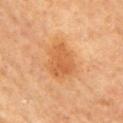This lesion was catalogued during total-body skin photography and was not selected for biopsy. This image is a 15 mm lesion crop taken from a total-body photograph. A female patient approximately 65 years of age. On the left arm. The tile uses cross-polarized illumination. Automated tile analysis of the lesion measured a border-irregularity index near 3/10, a within-lesion color-variation index near 3/10, and a peripheral color-asymmetry measure near 1. The software also gave a classifier nevus-likeness of about 95/100 and a lesion-detection confidence of about 100/100. Measured at roughly 4 mm in maximum diameter.